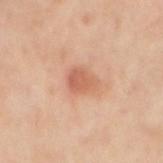The lesion was photographed on a routine skin check and not biopsied; there is no pathology result. A 15 mm close-up tile from a total-body photography series done for melanoma screening. Approximately 3 mm at its widest. The patient is a male aged 48–52. The lesion is on the left arm. The tile uses cross-polarized illumination.